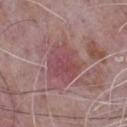• workup: total-body-photography surveillance lesion; no biopsy
• lesion diameter: about 5 mm
• image source: ~15 mm tile from a whole-body skin photo
• body site: the front of the torso
• patient: male, aged 63–67
• illumination: white-light